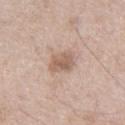Clinical impression:
Part of a total-body skin-imaging series; this lesion was reviewed on a skin check and was not flagged for biopsy.